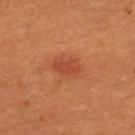{"biopsy_status": "not biopsied; imaged during a skin examination", "patient": {"sex": "female", "age_approx": 50}, "lesion_size": {"long_diameter_mm_approx": 3.0}, "lighting": "cross-polarized", "image": {"source": "total-body photography crop", "field_of_view_mm": 15}}